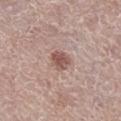  biopsy_status: not biopsied; imaged during a skin examination
  patient:
    sex: female
    age_approx: 65
  site: leg
  lesion_size:
    long_diameter_mm_approx: 2.5
  lighting: white-light
  image:
    source: total-body photography crop
    field_of_view_mm: 15